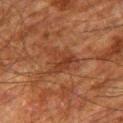| feature | finding |
|---|---|
| notes | catalogued during a skin exam; not biopsied |
| illumination | cross-polarized illumination |
| image source | ~15 mm tile from a whole-body skin photo |
| TBP lesion metrics | an eccentricity of roughly 0.45 |
| patient | male, aged 78 to 82 |
| body site | the right thigh |